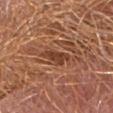This lesion was catalogued during total-body skin photography and was not selected for biopsy.
The lesion is located on the front of the torso.
A close-up tile cropped from a whole-body skin photograph, about 15 mm across.
The subject is a female approximately 45 years of age.
The lesion's longest dimension is about 4 mm.